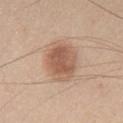workup = no biopsy performed (imaged during a skin exam) | patient = male, aged 63–67 | acquisition = total-body-photography crop, ~15 mm field of view | diameter = ≈5.5 mm | tile lighting = white-light | anatomic site = the chest.